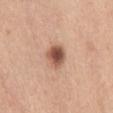| field | value |
|---|---|
| notes | catalogued during a skin exam; not biopsied |
| patient | female, aged 28–32 |
| imaging modality | total-body-photography crop, ~15 mm field of view |
| diameter | ≈3 mm |
| body site | the chest |